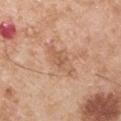Q: Was this lesion biopsied?
A: catalogued during a skin exam; not biopsied
Q: Lesion size?
A: ≈4.5 mm
Q: Where on the body is the lesion?
A: the left upper arm
Q: How was this image acquired?
A: 15 mm crop, total-body photography
Q: Illumination type?
A: white-light illumination
Q: What are the patient's age and sex?
A: male, aged 53–57
Q: Automated lesion metrics?
A: a border-irregularity index near 4.5/10, internal color variation of about 2.5 on a 0–10 scale, and radial color variation of about 0.5